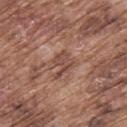<lesion>
  <biopsy_status>not biopsied; imaged during a skin examination</biopsy_status>
  <lighting>white-light</lighting>
  <site>upper back</site>
  <patient>
    <sex>male</sex>
    <age_approx>75</age_approx>
  </patient>
  <image>
    <source>total-body photography crop</source>
    <field_of_view_mm>15</field_of_view_mm>
  </image>
</lesion>